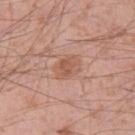{"image": {"source": "total-body photography crop", "field_of_view_mm": 15}, "site": "right thigh", "patient": {"sex": "male", "age_approx": 55}, "automated_metrics": {"eccentricity": 0.7, "shape_asymmetry": 0.2, "cielab_L": 56, "cielab_a": 22, "cielab_b": 30, "vs_skin_darker_L": 8.0, "vs_skin_contrast_norm": 6.0, "border_irregularity_0_10": 2.0, "color_variation_0_10": 2.5}}